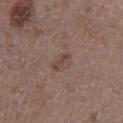{"site": "left lower leg", "lesion_size": {"long_diameter_mm_approx": 2.5}, "image": {"source": "total-body photography crop", "field_of_view_mm": 15}, "automated_metrics": {"border_irregularity_0_10": 4.0, "peripheral_color_asymmetry": 0.0, "nevus_likeness_0_100": 0, "lesion_detection_confidence_0_100": 100}, "patient": {"sex": "male", "age_approx": 45}}